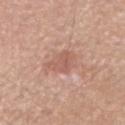The lesion was tiled from a total-body skin photograph and was not biopsied.
Measured at roughly 4.5 mm in maximum diameter.
A 15 mm close-up tile from a total-body photography series done for melanoma screening.
The patient is a male about 75 years old.
Located on the right forearm.
An algorithmic analysis of the crop reported a lesion area of about 8 mm², an eccentricity of roughly 0.8, and two-axis asymmetry of about 0.3. And it measured an average lesion color of about L≈58 a*≈22 b*≈27 (CIELAB), a lesion–skin lightness drop of about 8, and a lesion-to-skin contrast of about 5.5 (normalized; higher = more distinct). The software also gave a border-irregularity rating of about 3.5/10, a within-lesion color-variation index near 2/10, and a peripheral color-asymmetry measure near 1. And it measured a classifier nevus-likeness of about 0/100.